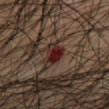Imaged during a routine full-body skin examination; the lesion was not biopsied and no histopathology is available. Located on the abdomen. The patient is a male about 50 years old. A 15 mm crop from a total-body photograph taken for skin-cancer surveillance. The total-body-photography lesion software estimated a lesion area of about 6 mm². The software also gave a border-irregularity index near 4.5/10, a color-variation rating of about 6/10, and radial color variation of about 2. The software also gave a classifier nevus-likeness of about 0/100 and a detector confidence of about 100 out of 100 that the crop contains a lesion. Measured at roughly 3.5 mm in maximum diameter. This is a cross-polarized tile.